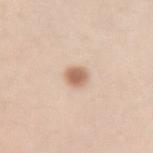Impression:
The lesion was tiled from a total-body skin photograph and was not biopsied.
Context:
On the left forearm. The subject is a female aged approximately 25. A 15 mm crop from a total-body photograph taken for skin-cancer surveillance.